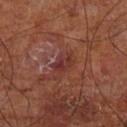acquisition: ~15 mm tile from a whole-body skin photo
lesion size: ≈2.5 mm
tile lighting: cross-polarized
body site: the left lower leg
subject: male, aged around 70